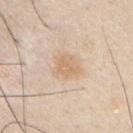Case summary:
* biopsy status — total-body-photography surveillance lesion; no biopsy
* site — the chest
* subject — male, in their 30s
* lighting — white-light
* automated lesion analysis — a mean CIELAB color near L≈69 a*≈15 b*≈33 and a normalized border contrast of about 5.5; a nevus-likeness score of about 10/100 and a detector confidence of about 100 out of 100 that the crop contains a lesion
* acquisition — ~15 mm crop, total-body skin-cancer survey